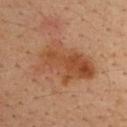Notes:
- biopsy status — imaged on a skin check; not biopsied
- diameter — ≈8 mm
- patient — male, aged 33–37
- lighting — cross-polarized
- imaging modality — total-body-photography crop, ~15 mm field of view
- body site — the upper back
- automated lesion analysis — a within-lesion color-variation index near 6/10 and radial color variation of about 2.5